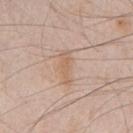<lesion>
  <biopsy_status>not biopsied; imaged during a skin examination</biopsy_status>
  <site>chest</site>
  <lighting>white-light</lighting>
  <lesion_size>
    <long_diameter_mm_approx>4.0</long_diameter_mm_approx>
  </lesion_size>
  <patient>
    <sex>male</sex>
    <age_approx>45</age_approx>
  </patient>
  <image>
    <source>total-body photography crop</source>
    <field_of_view_mm>15</field_of_view_mm>
  </image>
</lesion>